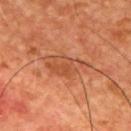{
  "biopsy_status": "not biopsied; imaged during a skin examination"
}